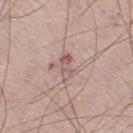Notes:
– notes: no biopsy performed (imaged during a skin exam)
– automated lesion analysis: a mean CIELAB color near L≈57 a*≈20 b*≈21, about 9 CIELAB-L* units darker than the surrounding skin, and a lesion-to-skin contrast of about 6.5 (normalized; higher = more distinct)
– location: the left thigh
– image source: total-body-photography crop, ~15 mm field of view
– subject: male, aged 58–62
– size: about 3 mm